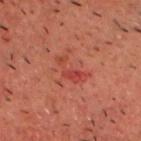Q: What lighting was used for the tile?
A: cross-polarized
Q: What is the lesion's diameter?
A: ~4 mm (longest diameter)
Q: What are the patient's age and sex?
A: male, about 60 years old
Q: What is the imaging modality?
A: ~15 mm tile from a whole-body skin photo
Q: What is the anatomic site?
A: the head or neck